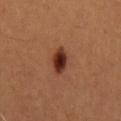No biopsy was performed on this lesion — it was imaged during a full skin examination and was not determined to be concerning.
A 15 mm crop from a total-body photograph taken for skin-cancer surveillance.
Longest diameter approximately 3.5 mm.
The subject is a male aged 63–67.
Automated tile analysis of the lesion measured about 14 CIELAB-L* units darker than the surrounding skin. It also reported a border-irregularity rating of about 2.5/10 and a peripheral color-asymmetry measure near 1.5. It also reported an automated nevus-likeness rating near 100 out of 100 and lesion-presence confidence of about 100/100.
From the mid back.